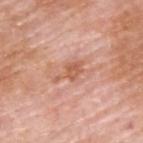Impression: Part of a total-body skin-imaging series; this lesion was reviewed on a skin check and was not flagged for biopsy. Background: The lesion's longest dimension is about 4 mm. Automated tile analysis of the lesion measured an area of roughly 4.5 mm² and an eccentricity of roughly 0.9. It also reported about 10 CIELAB-L* units darker than the surrounding skin. A 15 mm close-up tile from a total-body photography series done for melanoma screening. The subject is a male approximately 60 years of age. Located on the upper back.